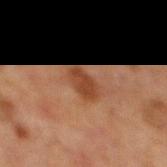{
  "biopsy_status": "not biopsied; imaged during a skin examination"
}